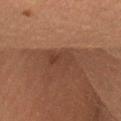Assessment:
The lesion was photographed on a routine skin check and not biopsied; there is no pathology result.
Image and clinical context:
The lesion is on the leg. A close-up tile cropped from a whole-body skin photograph, about 15 mm across. An algorithmic analysis of the crop reported an area of roughly 6.5 mm², an eccentricity of roughly 0.8, and a symmetry-axis asymmetry near 0.3. The analysis additionally found a border-irregularity index near 3.5/10 and a within-lesion color-variation index near 3/10. The patient is a female aged around 50.